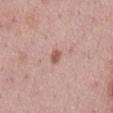<tbp_lesion>
<biopsy_status>not biopsied; imaged during a skin examination</biopsy_status>
<lesion_size>
  <long_diameter_mm_approx>2.0</long_diameter_mm_approx>
</lesion_size>
<site>back</site>
<lighting>white-light</lighting>
<image>
  <source>total-body photography crop</source>
  <field_of_view_mm>15</field_of_view_mm>
</image>
<patient>
  <sex>male</sex>
  <age_approx>45</age_approx>
</patient>
<automated_metrics>
  <border_irregularity_0_10>2.5</border_irregularity_0_10>
  <color_variation_0_10>0.0</color_variation_0_10>
  <peripheral_color_asymmetry>0.0</peripheral_color_asymmetry>
</automated_metrics>
</tbp_lesion>